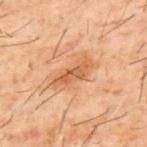  biopsy_status: not biopsied; imaged during a skin examination
  site: upper back
  lighting: cross-polarized
  lesion_size:
    long_diameter_mm_approx: 5.0
  image:
    source: total-body photography crop
    field_of_view_mm: 15
  patient:
    sex: male
    age_approx: 60